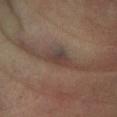Captured during whole-body skin photography for melanoma surveillance; the lesion was not biopsied. The patient is a male aged 63–67. The lesion is on the right forearm. A region of skin cropped from a whole-body photographic capture, roughly 15 mm wide.